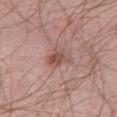<tbp_lesion>
  <biopsy_status>not biopsied; imaged during a skin examination</biopsy_status>
  <image>
    <source>total-body photography crop</source>
    <field_of_view_mm>15</field_of_view_mm>
  </image>
  <automated_metrics>
    <cielab_L>51</cielab_L>
    <cielab_a>22</cielab_a>
    <cielab_b>24</cielab_b>
    <vs_skin_contrast_norm>7.5</vs_skin_contrast_norm>
    <border_irregularity_0_10>3.5</border_irregularity_0_10>
    <color_variation_0_10>5.0</color_variation_0_10>
    <peripheral_color_asymmetry>1.5</peripheral_color_asymmetry>
    <nevus_likeness_0_100>50</nevus_likeness_0_100>
    <lesion_detection_confidence_0_100>100</lesion_detection_confidence_0_100>
  </automated_metrics>
  <site>left thigh</site>
  <lighting>white-light</lighting>
  <lesion_size>
    <long_diameter_mm_approx>3.0</long_diameter_mm_approx>
  </lesion_size>
  <patient>
    <sex>male</sex>
    <age_approx>55</age_approx>
  </patient>
</tbp_lesion>